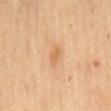Recorded during total-body skin imaging; not selected for excision or biopsy. The lesion is located on the mid back. Captured under cross-polarized illumination. A female patient aged approximately 70. A 15 mm close-up tile from a total-body photography series done for melanoma screening.